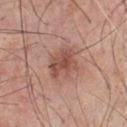Part of a total-body skin-imaging series; this lesion was reviewed on a skin check and was not flagged for biopsy. The patient is a male about 55 years old. On the chest. This image is a 15 mm lesion crop taken from a total-body photograph. Automated image analysis of the tile measured an area of roughly 10 mm², a shape eccentricity near 0.6, and a symmetry-axis asymmetry near 0.2. And it measured an average lesion color of about L≈51 a*≈22 b*≈27 (CIELAB), about 10 CIELAB-L* units darker than the surrounding skin, and a normalized lesion–skin contrast near 7.5. The analysis additionally found a border-irregularity rating of about 2.5/10, a within-lesion color-variation index near 4/10, and a peripheral color-asymmetry measure near 1.5. It also reported a classifier nevus-likeness of about 45/100. Captured under white-light illumination.